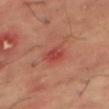| field | value |
|---|---|
| follow-up | catalogued during a skin exam; not biopsied |
| image | 15 mm crop, total-body photography |
| subject | male, approximately 65 years of age |
| location | the right thigh |